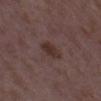The lesion was tiled from a total-body skin photograph and was not biopsied.
The lesion is on the left thigh.
Automated image analysis of the tile measured an average lesion color of about L≈31 a*≈16 b*≈19 (CIELAB), roughly 8 lightness units darker than nearby skin, and a lesion-to-skin contrast of about 8 (normalized; higher = more distinct). The analysis additionally found border irregularity of about 2 on a 0–10 scale, internal color variation of about 2 on a 0–10 scale, and peripheral color asymmetry of about 1. The software also gave lesion-presence confidence of about 100/100.
The subject is a female aged 33–37.
The tile uses white-light illumination.
A lesion tile, about 15 mm wide, cut from a 3D total-body photograph.